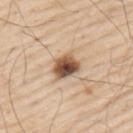Notes:
* subject: male, aged 78–82
* site: the upper back
* acquisition: ~15 mm crop, total-body skin-cancer survey